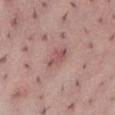Findings:
• follow-up: catalogued during a skin exam; not biopsied
• tile lighting: white-light illumination
• lesion diameter: about 3 mm
• site: the abdomen
• subject: female, aged 23 to 27
• image source: total-body-photography crop, ~15 mm field of view